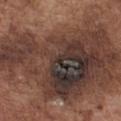biopsy_status: not biopsied; imaged during a skin examination
patient:
  sex: male
  age_approx: 75
lesion_size:
  long_diameter_mm_approx: 16.5
lighting: white-light
site: chest
image:
  source: total-body photography crop
  field_of_view_mm: 15